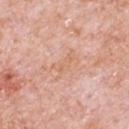Clinical impression:
Captured during whole-body skin photography for melanoma surveillance; the lesion was not biopsied.
Acquisition and patient details:
The lesion is on the front of the torso. Cropped from a total-body skin-imaging series; the visible field is about 15 mm. The patient is a male aged around 80.